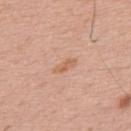Part of a total-body skin-imaging series; this lesion was reviewed on a skin check and was not flagged for biopsy. This image is a 15 mm lesion crop taken from a total-body photograph. Approximately 3 mm at its widest. The tile uses white-light illumination. A male patient about 55 years old. Automated image analysis of the tile measured a lesion area of about 3 mm² and an eccentricity of roughly 0.9. It also reported an average lesion color of about L≈62 a*≈22 b*≈33 (CIELAB) and a lesion-to-skin contrast of about 6 (normalized; higher = more distinct). The software also gave a classifier nevus-likeness of about 0/100.